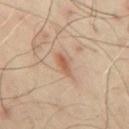  biopsy_status: not biopsied; imaged during a skin examination
  lesion_size:
    long_diameter_mm_approx: 3.5
  site: chest
  automated_metrics:
    area_mm2_approx: 3.5
    shape_asymmetry: 0.25
  image:
    source: total-body photography crop
    field_of_view_mm: 15
  patient:
    sex: male
    age_approx: 50